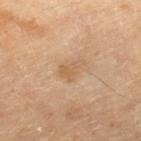Findings:
* subject · female, aged around 70
* location · the leg
* imaging modality · ~15 mm tile from a whole-body skin photo